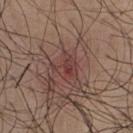Impression: The lesion was tiled from a total-body skin photograph and was not biopsied. Acquisition and patient details: The lesion's longest dimension is about 3 mm. A male subject, in their mid-20s. Located on the chest. A roughly 15 mm field-of-view crop from a total-body skin photograph. Captured under white-light illumination.